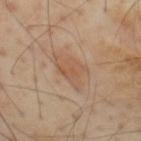Assessment:
Part of a total-body skin-imaging series; this lesion was reviewed on a skin check and was not flagged for biopsy.
Clinical summary:
On the upper back. A male subject aged 53–57. The tile uses cross-polarized illumination. A roughly 15 mm field-of-view crop from a total-body skin photograph.